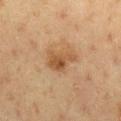Assessment: Imaged during a routine full-body skin examination; the lesion was not biopsied and no histopathology is available. Acquisition and patient details: Approximately 3.5 mm at its widest. On the chest. The patient is a male about 60 years old. A 15 mm close-up tile from a total-body photography series done for melanoma screening.